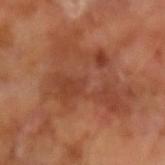The lesion was photographed on a routine skin check and not biopsied; there is no pathology result. This image is a 15 mm lesion crop taken from a total-body photograph. The subject is a male aged 63 to 67.